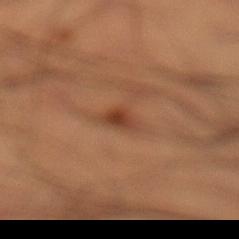Q: Is there a histopathology result?
A: imaged on a skin check; not biopsied
Q: What is the lesion's diameter?
A: about 3 mm
Q: Automated lesion metrics?
A: a mean CIELAB color near L≈32 a*≈19 b*≈26, about 8 CIELAB-L* units darker than the surrounding skin, and a lesion-to-skin contrast of about 7.5 (normalized; higher = more distinct); a border-irregularity index near 3.5/10, internal color variation of about 2.5 on a 0–10 scale, and peripheral color asymmetry of about 1; a nevus-likeness score of about 75/100 and lesion-presence confidence of about 100/100
Q: Where on the body is the lesion?
A: the left lower leg
Q: How was this image acquired?
A: ~15 mm crop, total-body skin-cancer survey
Q: Patient demographics?
A: male, about 50 years old